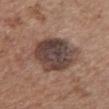Located on the chest. Automated tile analysis of the lesion measured a normalized border contrast of about 11.5. And it measured a color-variation rating of about 6.5/10. And it measured a nevus-likeness score of about 10/100. Imaged with white-light lighting. A female subject, roughly 75 years of age. A region of skin cropped from a whole-body photographic capture, roughly 15 mm wide. About 6 mm across.